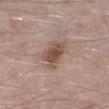A 15 mm crop from a total-body photograph taken for skin-cancer surveillance.
The tile uses white-light illumination.
The lesion is located on the left lower leg.
The patient is a male aged 38 to 42.